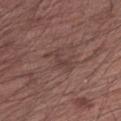This lesion was catalogued during total-body skin photography and was not selected for biopsy.
From the left upper arm.
Approximately 3.5 mm at its widest.
The tile uses white-light illumination.
The patient is a male in their mid- to late 60s.
A close-up tile cropped from a whole-body skin photograph, about 15 mm across.